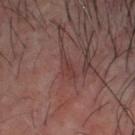| feature | finding |
|---|---|
| patient | male, approximately 60 years of age |
| lighting | cross-polarized illumination |
| body site | the head or neck |
| imaging modality | 15 mm crop, total-body photography |
| size | about 3.5 mm |